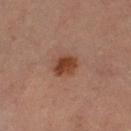This lesion was catalogued during total-body skin photography and was not selected for biopsy. An algorithmic analysis of the crop reported an average lesion color of about L≈35 a*≈21 b*≈28 (CIELAB) and a normalized border contrast of about 10. It also reported a border-irregularity index near 2/10 and a color-variation rating of about 3/10. The analysis additionally found a detector confidence of about 100 out of 100 that the crop contains a lesion. This is a cross-polarized tile. The subject is a female aged 68–72. About 3 mm across. A 15 mm crop from a total-body photograph taken for skin-cancer surveillance. On the left lower leg.